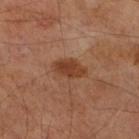biopsy status = catalogued during a skin exam; not biopsied | anatomic site = the right lower leg | lighting = cross-polarized | lesion diameter = ≈4 mm | image source = ~15 mm crop, total-body skin-cancer survey | patient = male, about 70 years old | automated lesion analysis = a lesion area of about 6.5 mm², an eccentricity of roughly 0.8, and two-axis asymmetry of about 0.2; a mean CIELAB color near L≈39 a*≈23 b*≈32, about 9 CIELAB-L* units darker than the surrounding skin, and a normalized lesion–skin contrast near 8; a border-irregularity rating of about 2.5/10, internal color variation of about 2.5 on a 0–10 scale, and peripheral color asymmetry of about 1.